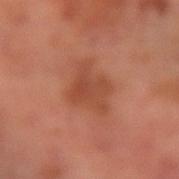Background:
This is a cross-polarized tile. Located on the right forearm. A close-up tile cropped from a whole-body skin photograph, about 15 mm across. The lesion's longest dimension is about 5 mm. A male subject, aged 68 to 72.